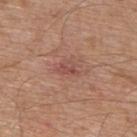{"biopsy_status": "not biopsied; imaged during a skin examination", "image": {"source": "total-body photography crop", "field_of_view_mm": 15}, "automated_metrics": {"area_mm2_approx": 3.5, "eccentricity": 0.75, "color_variation_0_10": 3.0, "lesion_detection_confidence_0_100": 100}, "patient": {"sex": "male", "age_approx": 65}, "lesion_size": {"long_diameter_mm_approx": 2.5}, "lighting": "white-light", "site": "upper back"}